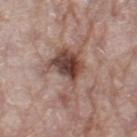Recorded during total-body skin imaging; not selected for excision or biopsy.
Cropped from a whole-body photographic skin survey; the tile spans about 15 mm.
Measured at roughly 9 mm in maximum diameter.
This is a white-light tile.
The patient is a female roughly 70 years of age.
The lesion is on the left thigh.